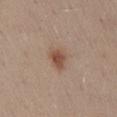The lesion was tiled from a total-body skin photograph and was not biopsied. Imaged with white-light lighting. The lesion is located on the mid back. Cropped from a total-body skin-imaging series; the visible field is about 15 mm. The patient is a female in their mid-40s. The total-body-photography lesion software estimated a border-irregularity index near 2.5/10, a within-lesion color-variation index near 3/10, and radial color variation of about 1. The software also gave an automated nevus-likeness rating near 100 out of 100 and a detector confidence of about 100 out of 100 that the crop contains a lesion.